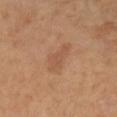biopsy_status: not biopsied; imaged during a skin examination
patient:
  sex: female
  age_approx: 65
image:
  source: total-body photography crop
  field_of_view_mm: 15
automated_metrics:
  area_mm2_approx: 7.0
  cielab_L: 52
  cielab_a: 21
  cielab_b: 32
  vs_skin_darker_L: 6.0
  vs_skin_contrast_norm: 4.5
  peripheral_color_asymmetry: 0.5
  nevus_likeness_0_100: 10
site: right forearm
lighting: cross-polarized
lesion_size:
  long_diameter_mm_approx: 4.0